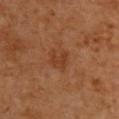Clinical impression: Recorded during total-body skin imaging; not selected for excision or biopsy. Context: About 2.5 mm across. Captured under cross-polarized illumination. A male patient, aged 58–62. A close-up tile cropped from a whole-body skin photograph, about 15 mm across. From the back. An algorithmic analysis of the crop reported a footprint of about 5 mm², a shape eccentricity near 0.45, and a shape-asymmetry score of about 0.3 (0 = symmetric). The software also gave about 6 CIELAB-L* units darker than the surrounding skin. And it measured a border-irregularity index near 3.5/10, a color-variation rating of about 1/10, and peripheral color asymmetry of about 0.5.